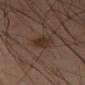This lesion was catalogued during total-body skin photography and was not selected for biopsy. Measured at roughly 3.5 mm in maximum diameter. A male patient aged around 55. A close-up tile cropped from a whole-body skin photograph, about 15 mm across. The tile uses cross-polarized illumination. Located on the left thigh.